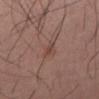  biopsy_status: not biopsied; imaged during a skin examination
  lighting: white-light
  image:
    source: total-body photography crop
    field_of_view_mm: 15
  lesion_size:
    long_diameter_mm_approx: 2.5
  site: right thigh
  patient:
    sex: male
    age_approx: 55